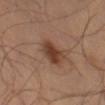Assessment:
The lesion was tiled from a total-body skin photograph and was not biopsied.
Background:
A 15 mm close-up extracted from a 3D total-body photography capture. The total-body-photography lesion software estimated a footprint of about 7.5 mm², a shape eccentricity near 0.75, and a symmetry-axis asymmetry near 0.2. It also reported an average lesion color of about L≈41 a*≈21 b*≈29 (CIELAB), a lesion–skin lightness drop of about 11, and a normalized border contrast of about 9. It also reported an automated nevus-likeness rating near 95 out of 100. From the left thigh. The recorded lesion diameter is about 3.5 mm.